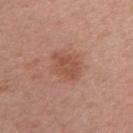Impression: The lesion was photographed on a routine skin check and not biopsied; there is no pathology result. Acquisition and patient details: From the right upper arm. A 15 mm close-up extracted from a 3D total-body photography capture. A female patient aged approximately 55. Measured at roughly 3.5 mm in maximum diameter. Captured under white-light illumination.